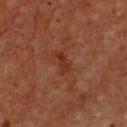Impression: No biopsy was performed on this lesion — it was imaged during a full skin examination and was not determined to be concerning. Context: The lesion is on the chest. Measured at roughly 3 mm in maximum diameter. A male patient, roughly 75 years of age. The lesion-visualizer software estimated an area of roughly 4 mm², a shape eccentricity near 0.85, and a shape-asymmetry score of about 0.3 (0 = symmetric). A region of skin cropped from a whole-body photographic capture, roughly 15 mm wide. Captured under cross-polarized illumination.